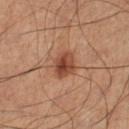* biopsy status: imaged on a skin check; not biopsied
* image-analysis metrics: a lesion area of about 6 mm², an eccentricity of roughly 0.6, and a shape-asymmetry score of about 0.15 (0 = symmetric)
* illumination: cross-polarized illumination
* acquisition: ~15 mm crop, total-body skin-cancer survey
* site: the leg
* diameter: about 3 mm
* subject: male, about 55 years old